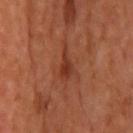Captured during whole-body skin photography for melanoma surveillance; the lesion was not biopsied.
Imaged with cross-polarized lighting.
A 15 mm crop from a total-body photograph taken for skin-cancer surveillance.
Located on the head or neck.
Automated image analysis of the tile measured a lesion area of about 4 mm², a shape eccentricity near 0.9, and a symmetry-axis asymmetry near 0.4. The software also gave a lesion color around L≈37 a*≈28 b*≈33 in CIELAB, about 9 CIELAB-L* units darker than the surrounding skin, and a normalized lesion–skin contrast near 7.5. The software also gave peripheral color asymmetry of about 1. And it measured a detector confidence of about 100 out of 100 that the crop contains a lesion.
A male patient, aged 63 to 67.
Measured at roughly 3.5 mm in maximum diameter.